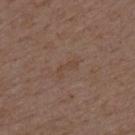| field | value |
|---|---|
| workup | no biopsy performed (imaged during a skin exam) |
| lesion size | ~3 mm (longest diameter) |
| location | the mid back |
| subject | male, aged 48 to 52 |
| acquisition | ~15 mm tile from a whole-body skin photo |
| illumination | white-light |